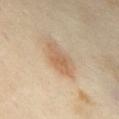Clinical impression:
Part of a total-body skin-imaging series; this lesion was reviewed on a skin check and was not flagged for biopsy.
Acquisition and patient details:
About 4.5 mm across. The subject is a female approximately 55 years of age. This is a cross-polarized tile. The lesion is located on the mid back. The total-body-photography lesion software estimated a mean CIELAB color near L≈62 a*≈18 b*≈34, about 9 CIELAB-L* units darker than the surrounding skin, and a lesion-to-skin contrast of about 6.5 (normalized; higher = more distinct). This image is a 15 mm lesion crop taken from a total-body photograph.